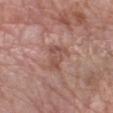Part of a total-body skin-imaging series; this lesion was reviewed on a skin check and was not flagged for biopsy. The lesion-visualizer software estimated an area of roughly 5.5 mm² and two-axis asymmetry of about 0.5. It also reported radial color variation of about 1. Imaged with white-light lighting. A female patient, roughly 70 years of age. Longest diameter approximately 3.5 mm. A 15 mm close-up extracted from a 3D total-body photography capture. The lesion is located on the arm.